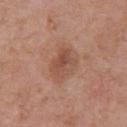Assessment:
Part of a total-body skin-imaging series; this lesion was reviewed on a skin check and was not flagged for biopsy.
Context:
A male subject, aged 68 to 72. Measured at roughly 4 mm in maximum diameter. A region of skin cropped from a whole-body photographic capture, roughly 15 mm wide. The lesion is on the chest. An algorithmic analysis of the crop reported a footprint of about 8 mm², an outline eccentricity of about 0.7 (0 = round, 1 = elongated), and two-axis asymmetry of about 0.25. The analysis additionally found a mean CIELAB color near L≈50 a*≈22 b*≈29 and roughly 8 lightness units darker than nearby skin. The software also gave a border-irregularity index near 2.5/10, internal color variation of about 5 on a 0–10 scale, and a peripheral color-asymmetry measure near 1.5. The analysis additionally found lesion-presence confidence of about 100/100. The tile uses white-light illumination.